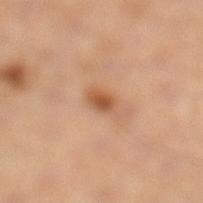Findings:
– follow-up — no biopsy performed (imaged during a skin exam)
– TBP lesion metrics — a lesion–skin lightness drop of about 11 and a normalized border contrast of about 8; a within-lesion color-variation index near 3.5/10; a classifier nevus-likeness of about 85/100 and lesion-presence confidence of about 100/100
– image source — ~15 mm crop, total-body skin-cancer survey
– lesion diameter — ≈2.5 mm
– anatomic site — the left leg
– subject — male, approximately 50 years of age
– lighting — cross-polarized